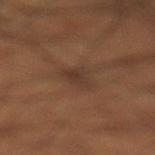Recorded during total-body skin imaging; not selected for excision or biopsy. On the left lower leg. The tile uses cross-polarized illumination. The lesion's longest dimension is about 3.5 mm. A male patient, aged approximately 50. Cropped from a total-body skin-imaging series; the visible field is about 15 mm.